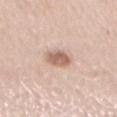Clinical impression: The lesion was photographed on a routine skin check and not biopsied; there is no pathology result. Image and clinical context: Captured under white-light illumination. A region of skin cropped from a whole-body photographic capture, roughly 15 mm wide. A female patient aged approximately 45. Located on the back.